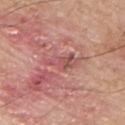Q: Is there a histopathology result?
A: imaged on a skin check; not biopsied
Q: Where on the body is the lesion?
A: the upper back
Q: What did automated image analysis measure?
A: a footprint of about 6 mm², an outline eccentricity of about 0.9 (0 = round, 1 = elongated), and two-axis asymmetry of about 0.35
Q: What are the patient's age and sex?
A: male, in their mid- to late 50s
Q: How large is the lesion?
A: ~4.5 mm (longest diameter)
Q: What kind of image is this?
A: total-body-photography crop, ~15 mm field of view
Q: How was the tile lit?
A: white-light